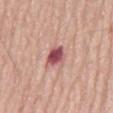Clinical impression: Imaged during a routine full-body skin examination; the lesion was not biopsied and no histopathology is available. Image and clinical context: A male subject aged 78–82. This is a white-light tile. The total-body-photography lesion software estimated a lesion area of about 5.5 mm², a shape eccentricity near 0.75, and two-axis asymmetry of about 0.2. The analysis additionally found border irregularity of about 2 on a 0–10 scale and radial color variation of about 1.5. The analysis additionally found an automated nevus-likeness rating near 15 out of 100 and a detector confidence of about 100 out of 100 that the crop contains a lesion. On the mid back. A lesion tile, about 15 mm wide, cut from a 3D total-body photograph.